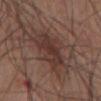An algorithmic analysis of the crop reported a lesion color around L≈35 a*≈18 b*≈22 in CIELAB, a lesion–skin lightness drop of about 7, and a normalized border contrast of about 7. And it measured border irregularity of about 4.5 on a 0–10 scale, internal color variation of about 3.5 on a 0–10 scale, and a peripheral color-asymmetry measure near 1. The lesion is on the abdomen. A close-up tile cropped from a whole-body skin photograph, about 15 mm across. This is a white-light tile. Measured at roughly 6 mm in maximum diameter. A male subject, aged 53–57.